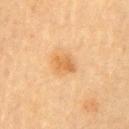The total-body-photography lesion software estimated a footprint of about 4.5 mm² and a shape-asymmetry score of about 0.25 (0 = symmetric). The software also gave an average lesion color of about L≈57 a*≈20 b*≈40 (CIELAB) and about 8 CIELAB-L* units darker than the surrounding skin. And it measured a border-irregularity rating of about 2.5/10, a within-lesion color-variation index near 1.5/10, and a peripheral color-asymmetry measure near 0.5. The software also gave a nevus-likeness score of about 75/100 and a detector confidence of about 100 out of 100 that the crop contains a lesion. A 15 mm close-up extracted from a 3D total-body photography capture. The lesion is located on the left upper arm. About 2.5 mm across. Imaged with cross-polarized lighting. A male patient aged approximately 85 or older.